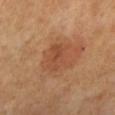Background: The patient is a male aged 63–67. On the left lower leg. A roughly 15 mm field-of-view crop from a total-body skin photograph. About 4 mm across. Automated tile analysis of the lesion measured a shape eccentricity near 0.35 and a shape-asymmetry score of about 0.3 (0 = symmetric). It also reported a mean CIELAB color near L≈46 a*≈22 b*≈33 and a normalized border contrast of about 5. The software also gave border irregularity of about 3.5 on a 0–10 scale, a color-variation rating of about 3.5/10, and a peripheral color-asymmetry measure near 1.5.